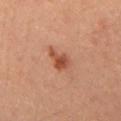{
  "biopsy_status": "not biopsied; imaged during a skin examination",
  "automated_metrics": {
    "area_mm2_approx": 5.0,
    "eccentricity": 0.7,
    "shape_asymmetry": 0.3,
    "cielab_L": 37,
    "cielab_a": 20,
    "cielab_b": 26,
    "vs_skin_darker_L": 9.0,
    "vs_skin_contrast_norm": 8.0,
    "border_irregularity_0_10": 3.0,
    "color_variation_0_10": 3.0,
    "peripheral_color_asymmetry": 1.0
  },
  "site": "abdomen",
  "patient": {
    "sex": "male",
    "age_approx": 50
  },
  "lighting": "cross-polarized",
  "lesion_size": {
    "long_diameter_mm_approx": 3.0
  },
  "image": {
    "source": "total-body photography crop",
    "field_of_view_mm": 15
  }
}